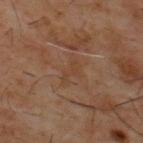Q: Was a biopsy performed?
A: total-body-photography surveillance lesion; no biopsy
Q: What is the lesion's diameter?
A: ≈3.5 mm
Q: How was the tile lit?
A: cross-polarized illumination
Q: Who is the patient?
A: male, approximately 60 years of age
Q: How was this image acquired?
A: ~15 mm crop, total-body skin-cancer survey
Q: What is the anatomic site?
A: the upper back